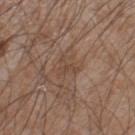biopsy status: no biopsy performed (imaged during a skin exam) | diameter: ≈2.5 mm | imaging modality: ~15 mm crop, total-body skin-cancer survey | illumination: white-light illumination | subject: male, in their mid- to late 50s | automated lesion analysis: a lesion area of about 2.5 mm² and an outline eccentricity of about 0.85 (0 = round, 1 = elongated); a mean CIELAB color near L≈45 a*≈16 b*≈26, a lesion–skin lightness drop of about 6, and a lesion-to-skin contrast of about 5 (normalized; higher = more distinct); a border-irregularity rating of about 6.5/10, a within-lesion color-variation index near 0/10, and radial color variation of about 0 | location: the right lower leg.